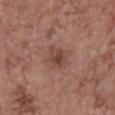biopsy status: total-body-photography surveillance lesion; no biopsy | subject: female, aged 63 to 67 | site: the chest | image: ~15 mm crop, total-body skin-cancer survey | lesion diameter: about 3 mm.